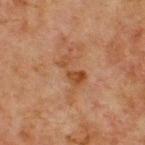Recorded during total-body skin imaging; not selected for excision or biopsy.
The lesion is on the chest.
A male patient, approximately 65 years of age.
The total-body-photography lesion software estimated a footprint of about 5 mm², an eccentricity of roughly 0.9, and two-axis asymmetry of about 0.45. The analysis additionally found an average lesion color of about L≈38 a*≈20 b*≈30 (CIELAB), about 7 CIELAB-L* units darker than the surrounding skin, and a lesion-to-skin contrast of about 7 (normalized; higher = more distinct). And it measured border irregularity of about 6 on a 0–10 scale and radial color variation of about 1. The software also gave a nevus-likeness score of about 0/100 and lesion-presence confidence of about 100/100.
A 15 mm close-up tile from a total-body photography series done for melanoma screening.
The recorded lesion diameter is about 3.5 mm.
The tile uses cross-polarized illumination.